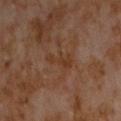Q: Was a biopsy performed?
A: imaged on a skin check; not biopsied
Q: What is the imaging modality?
A: ~15 mm crop, total-body skin-cancer survey
Q: What is the anatomic site?
A: the chest
Q: Lesion size?
A: ~4 mm (longest diameter)
Q: Automated lesion metrics?
A: a mean CIELAB color near L≈33 a*≈18 b*≈28 and a normalized border contrast of about 5.5; border irregularity of about 6 on a 0–10 scale and internal color variation of about 1 on a 0–10 scale; a lesion-detection confidence of about 100/100
Q: Patient demographics?
A: male, aged 58–62